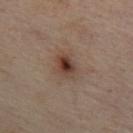Case summary:
• notes: no biopsy performed (imaged during a skin exam)
• body site: the chest
• image-analysis metrics: an area of roughly 5 mm², an eccentricity of roughly 0.65, and two-axis asymmetry of about 0.2; an average lesion color of about L≈31 a*≈15 b*≈21 (CIELAB), roughly 10 lightness units darker than nearby skin, and a normalized border contrast of about 9.5; an automated nevus-likeness rating near 90 out of 100 and a lesion-detection confidence of about 100/100
• patient: female, in their mid- to late 50s
• image source: total-body-photography crop, ~15 mm field of view